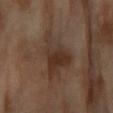<lesion>
<biopsy_status>not biopsied; imaged during a skin examination</biopsy_status>
<lesion_size>
  <long_diameter_mm_approx>7.0</long_diameter_mm_approx>
</lesion_size>
<site>left forearm</site>
<image>
  <source>total-body photography crop</source>
  <field_of_view_mm>15</field_of_view_mm>
</image>
<patient>
  <sex>female</sex>
  <age_approx>70</age_approx>
</patient>
<automated_metrics>
  <cielab_L>33</cielab_L>
  <cielab_a>16</cielab_a>
  <cielab_b>24</cielab_b>
  <vs_skin_darker_L>8.0</vs_skin_darker_L>
  <vs_skin_contrast_norm>8.0</vs_skin_contrast_norm>
  <border_irregularity_0_10>5.5</border_irregularity_0_10>
  <lesion_detection_confidence_0_100>95</lesion_detection_confidence_0_100>
</automated_metrics>
<lighting>cross-polarized</lighting>
</lesion>